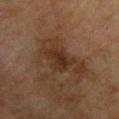notes = imaged on a skin check; not biopsied
subject = male, in their mid- to late 70s
image = ~15 mm crop, total-body skin-cancer survey
body site = the left upper arm